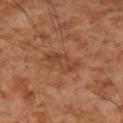The lesion was tiled from a total-body skin photograph and was not biopsied. A roughly 15 mm field-of-view crop from a total-body skin photograph. Located on the right upper arm. A male subject aged 53 to 57.